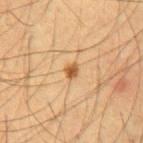This lesion was catalogued during total-body skin photography and was not selected for biopsy.
The lesion is on the abdomen.
The lesion-visualizer software estimated a footprint of about 2.5 mm², an outline eccentricity of about 0.6 (0 = round, 1 = elongated), and two-axis asymmetry of about 0.25. And it measured roughly 13 lightness units darker than nearby skin and a normalized border contrast of about 9. The analysis additionally found a color-variation rating of about 2.5/10.
A male patient aged approximately 55.
The recorded lesion diameter is about 2 mm.
A 15 mm close-up extracted from a 3D total-body photography capture.
Captured under cross-polarized illumination.